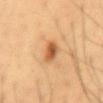Clinical impression:
Captured during whole-body skin photography for melanoma surveillance; the lesion was not biopsied.
Background:
A male subject, approximately 60 years of age. Automated image analysis of the tile measured an area of roughly 5.5 mm² and two-axis asymmetry of about 0.2. This is a cross-polarized tile. The lesion is on the front of the torso. A close-up tile cropped from a whole-body skin photograph, about 15 mm across. About 3.5 mm across.